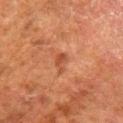Q: Was this lesion biopsied?
A: catalogued during a skin exam; not biopsied
Q: Lesion size?
A: ≈2.5 mm
Q: Automated lesion metrics?
A: a symmetry-axis asymmetry near 0.4; a lesion-to-skin contrast of about 6 (normalized; higher = more distinct); border irregularity of about 4 on a 0–10 scale, internal color variation of about 2 on a 0–10 scale, and radial color variation of about 0.5
Q: What lighting was used for the tile?
A: cross-polarized
Q: How was this image acquired?
A: total-body-photography crop, ~15 mm field of view
Q: Lesion location?
A: the leg
Q: Who is the patient?
A: male, aged 78 to 82